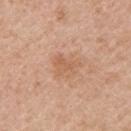Q: Was a biopsy performed?
A: catalogued during a skin exam; not biopsied
Q: Automated lesion metrics?
A: a footprint of about 6.5 mm², an eccentricity of roughly 0.6, and two-axis asymmetry of about 0.4; a mean CIELAB color near L≈60 a*≈21 b*≈34, about 7 CIELAB-L* units darker than the surrounding skin, and a normalized lesion–skin contrast near 5; border irregularity of about 4 on a 0–10 scale and a within-lesion color-variation index near 2/10; an automated nevus-likeness rating near 0 out of 100 and a lesion-detection confidence of about 100/100
Q: Lesion location?
A: the right upper arm
Q: How large is the lesion?
A: about 3.5 mm
Q: Who is the patient?
A: female, aged around 60
Q: How was this image acquired?
A: total-body-photography crop, ~15 mm field of view
Q: What lighting was used for the tile?
A: white-light